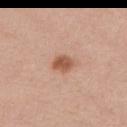{
  "biopsy_status": "not biopsied; imaged during a skin examination",
  "patient": {
    "sex": "female",
    "age_approx": 45
  },
  "lighting": "white-light",
  "lesion_size": {
    "long_diameter_mm_approx": 2.5
  },
  "site": "front of the torso",
  "image": {
    "source": "total-body photography crop",
    "field_of_view_mm": 15
  },
  "automated_metrics": {
    "eccentricity": 0.6,
    "shape_asymmetry": 0.2,
    "cielab_L": 56,
    "cielab_a": 23,
    "cielab_b": 32,
    "vs_skin_contrast_norm": 8.0,
    "border_irregularity_0_10": 1.5,
    "peripheral_color_asymmetry": 1.0
  }
}